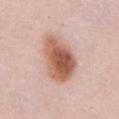- workup — total-body-photography surveillance lesion; no biopsy
- diameter — ≈6.5 mm
- location — the abdomen
- automated lesion analysis — a border-irregularity rating of about 2/10, a within-lesion color-variation index near 7.5/10, and peripheral color asymmetry of about 2.5; a nevus-likeness score of about 100/100
- tile lighting — white-light illumination
- acquisition — ~15 mm tile from a whole-body skin photo
- patient — female, aged approximately 40